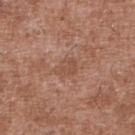Assessment: Recorded during total-body skin imaging; not selected for excision or biopsy. Image and clinical context: A lesion tile, about 15 mm wide, cut from a 3D total-body photograph. The lesion is on the upper back. The lesion's longest dimension is about 2.5 mm. A male patient, roughly 45 years of age. The total-body-photography lesion software estimated an area of roughly 4.5 mm², a shape eccentricity near 0.65, and a symmetry-axis asymmetry near 0.3. And it measured a normalized lesion–skin contrast near 5. The analysis additionally found a border-irregularity index near 2.5/10 and radial color variation of about 0.5. And it measured a nevus-likeness score of about 0/100. Captured under white-light illumination.